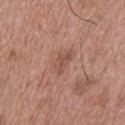Clinical impression:
Imaged during a routine full-body skin examination; the lesion was not biopsied and no histopathology is available.
Background:
About 3 mm across. The lesion is located on the upper back. A male subject in their mid- to late 60s. Automated image analysis of the tile measured a lesion area of about 3.5 mm², an eccentricity of roughly 0.85, and a symmetry-axis asymmetry near 0.4. A 15 mm close-up extracted from a 3D total-body photography capture.